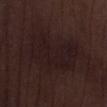The lesion was photographed on a routine skin check and not biopsied; there is no pathology result.